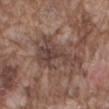Notes:
- notes · catalogued during a skin exam; not biopsied
- lighting · white-light
- subject · male, about 75 years old
- site · the mid back
- size · about 7.5 mm
- image · ~15 mm tile from a whole-body skin photo